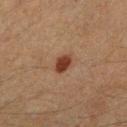Clinical impression:
No biopsy was performed on this lesion — it was imaged during a full skin examination and was not determined to be concerning.
Acquisition and patient details:
A male patient, aged 48 to 52. A roughly 15 mm field-of-view crop from a total-body skin photograph. Captured under cross-polarized illumination. On the right forearm. About 2.5 mm across. Automated tile analysis of the lesion measured a lesion color around L≈32 a*≈20 b*≈26 in CIELAB, roughly 11 lightness units darker than nearby skin, and a normalized lesion–skin contrast near 10.5. It also reported a border-irregularity index near 1.5/10, internal color variation of about 2 on a 0–10 scale, and radial color variation of about 0.5. The analysis additionally found an automated nevus-likeness rating near 100 out of 100 and a lesion-detection confidence of about 100/100.